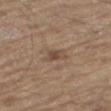Impression: The lesion was tiled from a total-body skin photograph and was not biopsied. Clinical summary: Approximately 2.5 mm at its widest. Cropped from a whole-body photographic skin survey; the tile spans about 15 mm. The subject is a male aged 68–72. The total-body-photography lesion software estimated an area of roughly 4 mm², a shape eccentricity near 0.8, and two-axis asymmetry of about 0.3. The software also gave border irregularity of about 2.5 on a 0–10 scale, a color-variation rating of about 3.5/10, and a peripheral color-asymmetry measure near 1. The tile uses white-light illumination. Located on the right thigh.